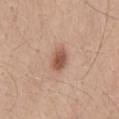Notes:
• workup · imaged on a skin check; not biopsied
• body site · the abdomen
• patient · male, aged approximately 30
• tile lighting · white-light
• imaging modality · 15 mm crop, total-body photography
• automated metrics · a border-irregularity rating of about 2/10, a within-lesion color-variation index near 3/10, and radial color variation of about 1; an automated nevus-likeness rating near 95 out of 100